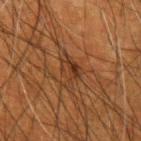patient = male, aged 48 to 52 | acquisition = ~15 mm crop, total-body skin-cancer survey | location = the arm.